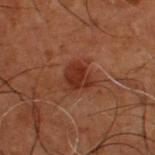Case summary:
• biopsy status · total-body-photography surveillance lesion; no biopsy
• acquisition · ~15 mm tile from a whole-body skin photo
• patient · male, aged 48 to 52
• site · the upper back